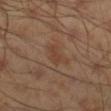{
  "biopsy_status": "not biopsied; imaged during a skin examination",
  "site": "right lower leg",
  "lesion_size": {
    "long_diameter_mm_approx": 2.5
  },
  "image": {
    "source": "total-body photography crop",
    "field_of_view_mm": 15
  },
  "automated_metrics": {
    "cielab_L": 40,
    "cielab_a": 19,
    "cielab_b": 28,
    "vs_skin_darker_L": 5.0,
    "vs_skin_contrast_norm": 5.0,
    "nevus_likeness_0_100": 5,
    "lesion_detection_confidence_0_100": 100
  },
  "patient": {
    "sex": "male",
    "age_approx": 45
  }
}